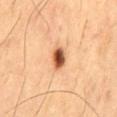Q: Automated lesion metrics?
A: a border-irregularity rating of about 2.5/10, internal color variation of about 8 on a 0–10 scale, and a peripheral color-asymmetry measure near 2.5; an automated nevus-likeness rating near 100 out of 100 and lesion-presence confidence of about 100/100
Q: What kind of image is this?
A: ~15 mm tile from a whole-body skin photo
Q: Illumination type?
A: cross-polarized illumination
Q: What are the patient's age and sex?
A: male, aged approximately 60
Q: How large is the lesion?
A: about 3 mm
Q: Lesion location?
A: the mid back